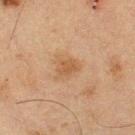biopsy status = no biopsy performed (imaged during a skin exam) | imaging modality = ~15 mm crop, total-body skin-cancer survey | illumination = cross-polarized | subject = male, aged around 65 | location = the right thigh | lesion diameter = ~2.5 mm (longest diameter).